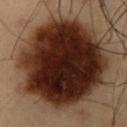workup: imaged on a skin check; not biopsied
illumination: cross-polarized
imaging modality: ~15 mm tile from a whole-body skin photo
subject: male, roughly 55 years of age
automated metrics: a lesion area of about 80 mm²; an average lesion color of about L≈19 a*≈16 b*≈20 (CIELAB), about 20 CIELAB-L* units darker than the surrounding skin, and a normalized lesion–skin contrast near 20
site: the abdomen
lesion size: ≈11.5 mm